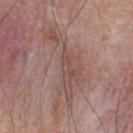Assessment: The lesion was tiled from a total-body skin photograph and was not biopsied. Acquisition and patient details: This is a white-light tile. Longest diameter approximately 9 mm. A male patient, roughly 65 years of age. Automated tile analysis of the lesion measured a lesion area of about 17 mm², an eccentricity of roughly 0.95, and two-axis asymmetry of about 0.7. It also reported a border-irregularity index near 10/10, a within-lesion color-variation index near 3/10, and a peripheral color-asymmetry measure near 1. And it measured a nevus-likeness score of about 0/100. A 15 mm close-up extracted from a 3D total-body photography capture. Located on the back.